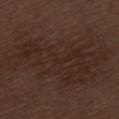No biopsy was performed on this lesion — it was imaged during a full skin examination and was not determined to be concerning. Approximately 14.5 mm at its widest. A male patient aged 68–72. Imaged with white-light lighting. The lesion is located on the left thigh. A close-up tile cropped from a whole-body skin photograph, about 15 mm across.